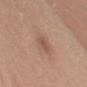• follow-up: catalogued during a skin exam; not biopsied
• site: the left upper arm
• imaging modality: ~15 mm tile from a whole-body skin photo
• subject: female, in their 40s
• diameter: ≈2.5 mm
• lighting: white-light illumination
• image-analysis metrics: an area of roughly 3 mm² and an outline eccentricity of about 0.7 (0 = round, 1 = elongated); a border-irregularity index near 2.5/10, a within-lesion color-variation index near 1.5/10, and a peripheral color-asymmetry measure near 0.5; a classifier nevus-likeness of about 0/100 and a lesion-detection confidence of about 100/100